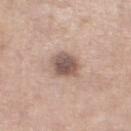biopsy_status: not biopsied; imaged during a skin examination
patient:
  sex: female
  age_approx: 55
image:
  source: total-body photography crop
  field_of_view_mm: 15
site: leg